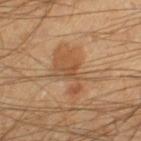| field | value |
|---|---|
| biopsy status | no biopsy performed (imaged during a skin exam) |
| imaging modality | 15 mm crop, total-body photography |
| size | ~2 mm (longest diameter) |
| location | the right lower leg |
| patient | male, aged approximately 45 |